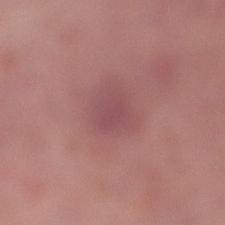biopsy status — catalogued during a skin exam; not biopsied
subject — female, aged 38–42
anatomic site — the right lower leg
tile lighting — white-light illumination
diameter — about 2.5 mm
image source — total-body-photography crop, ~15 mm field of view